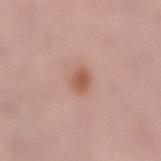Q: Who is the patient?
A: female, roughly 50 years of age
Q: How was this image acquired?
A: total-body-photography crop, ~15 mm field of view
Q: What lighting was used for the tile?
A: white-light illumination
Q: How large is the lesion?
A: ~2.5 mm (longest diameter)
Q: Lesion location?
A: the right lower leg